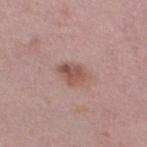follow-up: total-body-photography surveillance lesion; no biopsy.